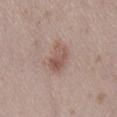The lesion was tiled from a total-body skin photograph and was not biopsied. Automated image analysis of the tile measured a lesion-detection confidence of about 100/100. Approximately 3.5 mm at its widest. The lesion is on the back. The subject is a female about 30 years old. A region of skin cropped from a whole-body photographic capture, roughly 15 mm wide.